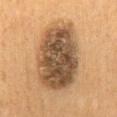Assessment: Captured during whole-body skin photography for melanoma surveillance; the lesion was not biopsied. Background: A lesion tile, about 15 mm wide, cut from a 3D total-body photograph. A male patient aged 58–62. From the mid back.